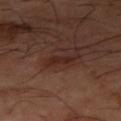<case>
  <lighting>cross-polarized</lighting>
  <patient>
    <sex>male</sex>
    <age_approx>65</age_approx>
  </patient>
  <image>
    <source>total-body photography crop</source>
    <field_of_view_mm>15</field_of_view_mm>
  </image>
  <site>right thigh</site>
  <lesion_size>
    <long_diameter_mm_approx>2.5</long_diameter_mm_approx>
  </lesion_size>
</case>